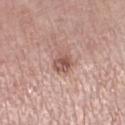Assessment:
The lesion was photographed on a routine skin check and not biopsied; there is no pathology result.
Acquisition and patient details:
Longest diameter approximately 2.5 mm. The total-body-photography lesion software estimated an average lesion color of about L≈54 a*≈21 b*≈24 (CIELAB), a lesion–skin lightness drop of about 11, and a lesion-to-skin contrast of about 7.5 (normalized; higher = more distinct). A female subject aged 68–72. The lesion is located on the left lower leg. This is a white-light tile. A lesion tile, about 15 mm wide, cut from a 3D total-body photograph.